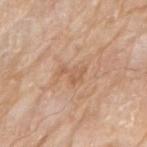{"biopsy_status": "not biopsied; imaged during a skin examination", "patient": {"sex": "male", "age_approx": 80}, "lesion_size": {"long_diameter_mm_approx": 3.0}, "site": "right upper arm", "image": {"source": "total-body photography crop", "field_of_view_mm": 15}, "automated_metrics": {"area_mm2_approx": 4.0, "shape_asymmetry": 0.65, "cielab_L": 59, "cielab_a": 20, "cielab_b": 33, "vs_skin_darker_L": 7.0, "vs_skin_contrast_norm": 5.0}, "lighting": "white-light"}